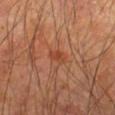| key | value |
|---|---|
| follow-up | total-body-photography surveillance lesion; no biopsy |
| lesion size | ~2.5 mm (longest diameter) |
| automated metrics | an outline eccentricity of about 0.8 (0 = round, 1 = elongated) and a shape-asymmetry score of about 0.3 (0 = symmetric); a border-irregularity rating of about 2.5/10, internal color variation of about 2 on a 0–10 scale, and peripheral color asymmetry of about 1; an automated nevus-likeness rating near 25 out of 100 and a lesion-detection confidence of about 100/100 |
| patient | male, aged 63–67 |
| image source | total-body-photography crop, ~15 mm field of view |
| lighting | cross-polarized |
| body site | the left forearm |